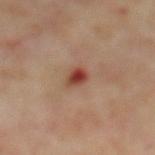Findings:
* follow-up: catalogued during a skin exam; not biopsied
* image: ~15 mm tile from a whole-body skin photo
* subject: male, aged approximately 70
* anatomic site: the mid back